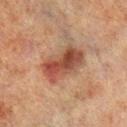Findings:
• notes — imaged on a skin check; not biopsied
• diameter — ≈6 mm
• location — the right lower leg
• illumination — cross-polarized illumination
• subject — male, approximately 70 years of age
• image — total-body-photography crop, ~15 mm field of view